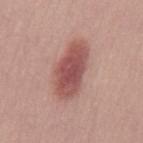follow-up = no biopsy performed (imaged during a skin exam); size = about 6.5 mm; subject = male, aged 28–32; lighting = white-light; imaging modality = 15 mm crop, total-body photography.